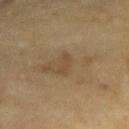This lesion was catalogued during total-body skin photography and was not selected for biopsy. Longest diameter approximately 3 mm. The lesion is on the right forearm. Imaged with cross-polarized lighting. Cropped from a total-body skin-imaging series; the visible field is about 15 mm. An algorithmic analysis of the crop reported a border-irregularity index near 5.5/10, a within-lesion color-variation index near 0/10, and peripheral color asymmetry of about 0. The patient is a female about 55 years old.